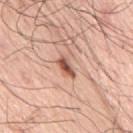Clinical impression:
Captured during whole-body skin photography for melanoma surveillance; the lesion was not biopsied.
Acquisition and patient details:
The lesion is on the mid back. Imaged with white-light lighting. A male patient roughly 75 years of age. Automated image analysis of the tile measured an area of roughly 3.5 mm². It also reported a classifier nevus-likeness of about 95/100 and lesion-presence confidence of about 100/100. A 15 mm close-up tile from a total-body photography series done for melanoma screening.